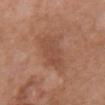{
  "biopsy_status": "not biopsied; imaged during a skin examination",
  "image": {
    "source": "total-body photography crop",
    "field_of_view_mm": 15
  },
  "patient": {
    "sex": "female",
    "age_approx": 70
  },
  "lesion_size": {
    "long_diameter_mm_approx": 5.0
  },
  "automated_metrics": {
    "area_mm2_approx": 14.0,
    "eccentricity": 0.8,
    "shape_asymmetry": 0.25
  },
  "site": "front of the torso"
}